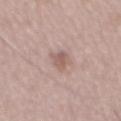{"biopsy_status": "not biopsied; imaged during a skin examination", "automated_metrics": {"vs_skin_darker_L": 9.0, "vs_skin_contrast_norm": 6.5}, "lesion_size": {"long_diameter_mm_approx": 3.0}, "lighting": "white-light", "patient": {"sex": "male", "age_approx": 55}, "image": {"source": "total-body photography crop", "field_of_view_mm": 15}}